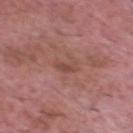Impression:
Recorded during total-body skin imaging; not selected for excision or biopsy.
Background:
Cropped from a whole-body photographic skin survey; the tile spans about 15 mm. Captured under white-light illumination. Approximately 3 mm at its widest. A male patient, aged 63 to 67. From the head or neck.